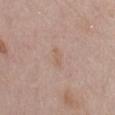Findings:
– biopsy status · catalogued during a skin exam; not biopsied